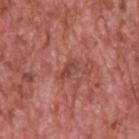{"biopsy_status": "not biopsied; imaged during a skin examination", "automated_metrics": {"vs_skin_darker_L": 8.0, "vs_skin_contrast_norm": 6.0, "nevus_likeness_0_100": 0, "lesion_detection_confidence_0_100": 100}, "image": {"source": "total-body photography crop", "field_of_view_mm": 15}, "patient": {"sex": "male", "age_approx": 60}, "lesion_size": {"long_diameter_mm_approx": 2.5}, "site": "head or neck"}